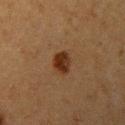The lesion was photographed on a routine skin check and not biopsied; there is no pathology result. Imaged with cross-polarized lighting. The patient is a female in their 40s. Cropped from a total-body skin-imaging series; the visible field is about 15 mm. Automated tile analysis of the lesion measured a lesion area of about 5 mm², an eccentricity of roughly 0.4, and two-axis asymmetry of about 0.2. It also reported an average lesion color of about L≈26 a*≈18 b*≈27 (CIELAB), roughly 10 lightness units darker than nearby skin, and a normalized border contrast of about 11. On the arm. About 2.5 mm across.